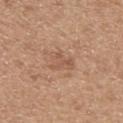biopsy_status: not biopsied; imaged during a skin examination
site: mid back
patient:
  sex: male
  age_approx: 65
lighting: white-light
lesion_size:
  long_diameter_mm_approx: 3.0
image:
  source: total-body photography crop
  field_of_view_mm: 15